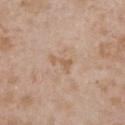Q: Is there a histopathology result?
A: no biopsy performed (imaged during a skin exam)
Q: Who is the patient?
A: female, approximately 30 years of age
Q: How large is the lesion?
A: ~3 mm (longest diameter)
Q: How was the tile lit?
A: white-light illumination
Q: What is the anatomic site?
A: the chest
Q: What is the imaging modality?
A: 15 mm crop, total-body photography